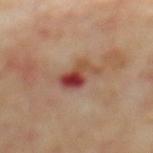| key | value |
|---|---|
| notes | no biopsy performed (imaged during a skin exam) |
| site | the mid back |
| diameter | ≈3.5 mm |
| subject | male, approximately 70 years of age |
| illumination | cross-polarized illumination |
| acquisition | total-body-photography crop, ~15 mm field of view |